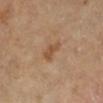| key | value |
|---|---|
| workup | no biopsy performed (imaged during a skin exam) |
| imaging modality | ~15 mm crop, total-body skin-cancer survey |
| image-analysis metrics | a mean CIELAB color near L≈51 a*≈20 b*≈33 and about 8 CIELAB-L* units darker than the surrounding skin; radial color variation of about 0.5 |
| patient | female, aged approximately 60 |
| anatomic site | the right lower leg |
| lesion diameter | ≈3 mm |